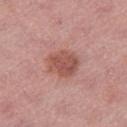No biopsy was performed on this lesion — it was imaged during a full skin examination and was not determined to be concerning.